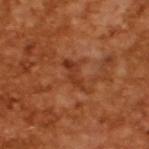<tbp_lesion>
<biopsy_status>not biopsied; imaged during a skin examination</biopsy_status>
<lighting>cross-polarized</lighting>
<image>
  <source>total-body photography crop</source>
  <field_of_view_mm>15</field_of_view_mm>
</image>
<patient>
  <sex>male</sex>
  <age_approx>65</age_approx>
</patient>
</tbp_lesion>